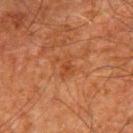{"patient": {"sex": "male", "age_approx": 80}, "image": {"source": "total-body photography crop", "field_of_view_mm": 15}, "lighting": "cross-polarized", "site": "mid back", "lesion_size": {"long_diameter_mm_approx": 2.5}}